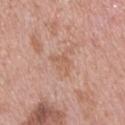| feature | finding |
|---|---|
| biopsy status | catalogued during a skin exam; not biopsied |
| diameter | ≈3 mm |
| lighting | white-light |
| image source | 15 mm crop, total-body photography |
| body site | the chest |
| automated metrics | a lesion–skin lightness drop of about 6 and a normalized border contrast of about 5; a classifier nevus-likeness of about 0/100 and a lesion-detection confidence of about 100/100 |
| patient | male, in their 50s |